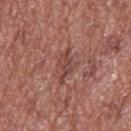<lesion>
<biopsy_status>not biopsied; imaged during a skin examination</biopsy_status>
<patient>
  <sex>male</sex>
  <age_approx>75</age_approx>
</patient>
<site>upper back</site>
<lighting>white-light</lighting>
<image>
  <source>total-body photography crop</source>
  <field_of_view_mm>15</field_of_view_mm>
</image>
<lesion_size>
  <long_diameter_mm_approx>4.0</long_diameter_mm_approx>
</lesion_size>
</lesion>